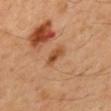biopsy status: total-body-photography surveillance lesion; no biopsy
anatomic site: the mid back
image-analysis metrics: a border-irregularity rating of about 2.5/10, a within-lesion color-variation index near 4/10, and peripheral color asymmetry of about 1.5; an automated nevus-likeness rating near 10 out of 100 and lesion-presence confidence of about 100/100
subject: male, aged around 45
tile lighting: cross-polarized
image source: ~15 mm tile from a whole-body skin photo
diameter: ~3 mm (longest diameter)